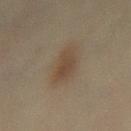• notes — total-body-photography surveillance lesion; no biopsy
• location — the mid back
• subject — female, aged around 45
• diameter — ≈4 mm
• acquisition — total-body-photography crop, ~15 mm field of view